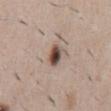This lesion was catalogued during total-body skin photography and was not selected for biopsy. The tile uses white-light illumination. On the abdomen. A male patient roughly 50 years of age. A close-up tile cropped from a whole-body skin photograph, about 15 mm across.